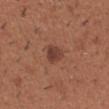Part of a total-body skin-imaging series; this lesion was reviewed on a skin check and was not flagged for biopsy. A male subject in their 40s. A 15 mm close-up extracted from a 3D total-body photography capture. The lesion is on the arm.